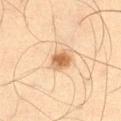No biopsy was performed on this lesion — it was imaged during a full skin examination and was not determined to be concerning.
Located on the abdomen.
An algorithmic analysis of the crop reported about 12 CIELAB-L* units darker than the surrounding skin and a normalized border contrast of about 8.5. The software also gave a border-irregularity rating of about 2/10, a within-lesion color-variation index near 3.5/10, and radial color variation of about 1. The analysis additionally found a detector confidence of about 100 out of 100 that the crop contains a lesion.
Longest diameter approximately 3 mm.
Captured under cross-polarized illumination.
A close-up tile cropped from a whole-body skin photograph, about 15 mm across.
A male subject, aged 63 to 67.